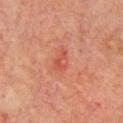Recorded during total-body skin imaging; not selected for excision or biopsy.
Cropped from a whole-body photographic skin survey; the tile spans about 15 mm.
The tile uses cross-polarized illumination.
The patient is a male approximately 65 years of age.
The lesion is located on the upper back.